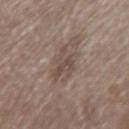acquisition: ~15 mm crop, total-body skin-cancer survey | body site: the left forearm | diameter: ≈3.5 mm | image-analysis metrics: a footprint of about 6.5 mm², an outline eccentricity of about 0.65 (0 = round, 1 = elongated), and a shape-asymmetry score of about 0.45 (0 = symmetric); an average lesion color of about L≈47 a*≈14 b*≈22 (CIELAB), about 8 CIELAB-L* units darker than the surrounding skin, and a normalized border contrast of about 6 | subject: female, approximately 50 years of age.